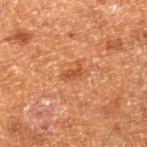This lesion was catalogued during total-body skin photography and was not selected for biopsy.
Imaged with cross-polarized lighting.
The lesion is located on the right lower leg.
About 2.5 mm across.
Automated image analysis of the tile measured a lesion color around L≈39 a*≈23 b*≈31 in CIELAB, a lesion–skin lightness drop of about 8, and a normalized lesion–skin contrast near 6.5. And it measured a detector confidence of about 100 out of 100 that the crop contains a lesion.
A male patient about 60 years old.
A 15 mm close-up extracted from a 3D total-body photography capture.